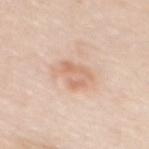Impression:
The lesion was tiled from a total-body skin photograph and was not biopsied.
Acquisition and patient details:
Cropped from a whole-body photographic skin survey; the tile spans about 15 mm. The lesion is on the mid back. A female patient, aged approximately 50.